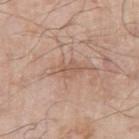acquisition: ~15 mm tile from a whole-body skin photo
anatomic site: the arm
subject: male, aged approximately 70
illumination: white-light illumination
size: about 4 mm